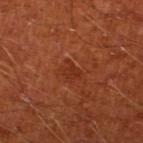Background:
The tile uses cross-polarized illumination. About 2.5 mm across. A lesion tile, about 15 mm wide, cut from a 3D total-body photograph. From the left lower leg. A male patient, aged approximately 65.